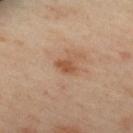Clinical impression:
No biopsy was performed on this lesion — it was imaged during a full skin examination and was not determined to be concerning.
Background:
Automated tile analysis of the lesion measured border irregularity of about 2.5 on a 0–10 scale and internal color variation of about 1.5 on a 0–10 scale. It also reported a nevus-likeness score of about 0/100 and lesion-presence confidence of about 100/100. The subject is a female about 40 years old. From the upper back. Imaged with cross-polarized lighting. This image is a 15 mm lesion crop taken from a total-body photograph. Approximately 2.5 mm at its widest.